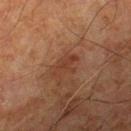<tbp_lesion>
<biopsy_status>not biopsied; imaged during a skin examination</biopsy_status>
<site>left thigh</site>
<image>
  <source>total-body photography crop</source>
  <field_of_view_mm>15</field_of_view_mm>
</image>
<lesion_size>
  <long_diameter_mm_approx>3.5</long_diameter_mm_approx>
</lesion_size>
<lighting>cross-polarized</lighting>
<patient>
  <sex>male</sex>
  <age_approx>80</age_approx>
</patient>
<automated_metrics>
  <area_mm2_approx>4.5</area_mm2_approx>
  <eccentricity>0.85</eccentricity>
  <shape_asymmetry>0.45</shape_asymmetry>
  <peripheral_color_asymmetry>0.5</peripheral_color_asymmetry>
</automated_metrics>
</tbp_lesion>